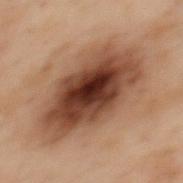Part of a total-body skin-imaging series; this lesion was reviewed on a skin check and was not flagged for biopsy.